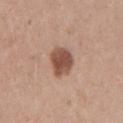Captured during whole-body skin photography for melanoma surveillance; the lesion was not biopsied. A 15 mm close-up extracted from a 3D total-body photography capture. Longest diameter approximately 3.5 mm. On the arm. Captured under white-light illumination. A male patient aged 23–27.